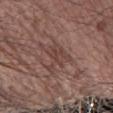Q: Where on the body is the lesion?
A: the right forearm
Q: Lesion size?
A: ≈5.5 mm
Q: What did automated image analysis measure?
A: border irregularity of about 8 on a 0–10 scale, a color-variation rating of about 3/10, and radial color variation of about 1; a nevus-likeness score of about 0/100 and a lesion-detection confidence of about 50/100
Q: Patient demographics?
A: male, aged approximately 65
Q: How was this image acquired?
A: 15 mm crop, total-body photography
Q: What lighting was used for the tile?
A: white-light